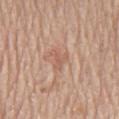This lesion was catalogued during total-body skin photography and was not selected for biopsy.
A 15 mm crop from a total-body photograph taken for skin-cancer surveillance.
A male subject aged around 80.
About 3 mm across.
An algorithmic analysis of the crop reported a color-variation rating of about 0/10 and peripheral color asymmetry of about 0. It also reported a nevus-likeness score of about 0/100.
The lesion is located on the chest.